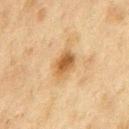Clinical impression: This lesion was catalogued during total-body skin photography and was not selected for biopsy. Clinical summary: Located on the chest. A 15 mm crop from a total-body photograph taken for skin-cancer surveillance. Automated image analysis of the tile measured a footprint of about 7 mm² and a shape-asymmetry score of about 0.15 (0 = symmetric). The software also gave a mean CIELAB color near L≈48 a*≈16 b*≈35, about 10 CIELAB-L* units darker than the surrounding skin, and a normalized lesion–skin contrast near 8.5. The analysis additionally found a nevus-likeness score of about 85/100 and a detector confidence of about 100 out of 100 that the crop contains a lesion. The tile uses cross-polarized illumination. A male patient, aged approximately 75. Longest diameter approximately 3.5 mm.